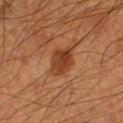Q: Was this lesion biopsied?
A: imaged on a skin check; not biopsied
Q: Patient demographics?
A: male, aged 53–57
Q: What is the lesion's diameter?
A: ≈3.5 mm
Q: How was this image acquired?
A: total-body-photography crop, ~15 mm field of view
Q: Automated lesion metrics?
A: an area of roughly 7.5 mm², an eccentricity of roughly 0.7, and two-axis asymmetry of about 0.2; a mean CIELAB color near L≈34 a*≈23 b*≈31 and about 9 CIELAB-L* units darker than the surrounding skin; a classifier nevus-likeness of about 80/100 and a detector confidence of about 100 out of 100 that the crop contains a lesion
Q: What is the anatomic site?
A: the arm
Q: How was the tile lit?
A: cross-polarized illumination